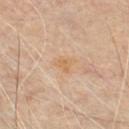Clinical impression: Part of a total-body skin-imaging series; this lesion was reviewed on a skin check and was not flagged for biopsy. Image and clinical context: About 2.5 mm across. A region of skin cropped from a whole-body photographic capture, roughly 15 mm wide. A male subject aged approximately 65. On the chest.